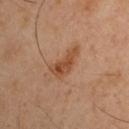{
  "biopsy_status": "not biopsied; imaged during a skin examination",
  "site": "left upper arm",
  "automated_metrics": {
    "area_mm2_approx": 6.0,
    "eccentricity": 0.9,
    "shape_asymmetry": 0.5,
    "cielab_L": 44,
    "cielab_a": 22,
    "cielab_b": 32,
    "vs_skin_darker_L": 9.0,
    "vs_skin_contrast_norm": 8.0,
    "lesion_detection_confidence_0_100": 100
  },
  "patient": {
    "sex": "male",
    "age_approx": 45
  },
  "lighting": "cross-polarized",
  "image": {
    "source": "total-body photography crop",
    "field_of_view_mm": 15
  },
  "lesion_size": {
    "long_diameter_mm_approx": 4.0
  }
}